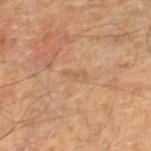biopsy_status: not biopsied; imaged during a skin examination
lesion_size:
  long_diameter_mm_approx: 2.5
site: right lower leg
image:
  source: total-body photography crop
  field_of_view_mm: 15
patient:
  sex: male
  age_approx: 55
lighting: cross-polarized